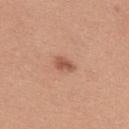Recorded during total-body skin imaging; not selected for excision or biopsy. A male subject, roughly 30 years of age. A close-up tile cropped from a whole-body skin photograph, about 15 mm across. Automated tile analysis of the lesion measured a shape eccentricity near 0.75 and a shape-asymmetry score of about 0.2 (0 = symmetric). It also reported a lesion color around L≈55 a*≈24 b*≈31 in CIELAB, roughly 11 lightness units darker than nearby skin, and a normalized lesion–skin contrast near 7.5. The lesion is on the upper back.